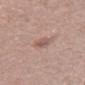Part of a total-body skin-imaging series; this lesion was reviewed on a skin check and was not flagged for biopsy.
Measured at roughly 3 mm in maximum diameter.
Automated tile analysis of the lesion measured a footprint of about 4.5 mm², an outline eccentricity of about 0.75 (0 = round, 1 = elongated), and two-axis asymmetry of about 0.2. The software also gave a border-irregularity rating of about 2/10 and internal color variation of about 3.5 on a 0–10 scale. The software also gave an automated nevus-likeness rating near 25 out of 100 and a detector confidence of about 100 out of 100 that the crop contains a lesion.
Captured under white-light illumination.
A 15 mm close-up extracted from a 3D total-body photography capture.
A male subject, aged around 75.
From the right thigh.